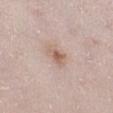Clinical impression:
Captured during whole-body skin photography for melanoma surveillance; the lesion was not biopsied.
Image and clinical context:
About 2.5 mm across. A female patient aged approximately 30. From the left thigh. Imaged with white-light lighting. A region of skin cropped from a whole-body photographic capture, roughly 15 mm wide.